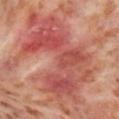Assessment:
This lesion was catalogued during total-body skin photography and was not selected for biopsy.
Clinical summary:
A region of skin cropped from a whole-body photographic capture, roughly 15 mm wide. The patient is a female aged around 55. Located on the right lower leg. This is a cross-polarized tile. An algorithmic analysis of the crop reported an average lesion color of about L≈53 a*≈33 b*≈28 (CIELAB) and roughly 10 lightness units darker than nearby skin. It also reported a border-irregularity index near 8.5/10, a color-variation rating of about 8/10, and radial color variation of about 2.5. The analysis additionally found a nevus-likeness score of about 0/100 and a detector confidence of about 70 out of 100 that the crop contains a lesion.